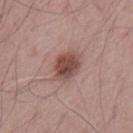The lesion was tiled from a total-body skin photograph and was not biopsied. A male subject, aged around 65. The total-body-photography lesion software estimated a footprint of about 10 mm², an eccentricity of roughly 0.7, and a shape-asymmetry score of about 0.2 (0 = symmetric). The software also gave an average lesion color of about L≈48 a*≈20 b*≈23 (CIELAB), roughly 13 lightness units darker than nearby skin, and a normalized lesion–skin contrast near 9. And it measured a border-irregularity rating of about 2/10, internal color variation of about 5 on a 0–10 scale, and a peripheral color-asymmetry measure near 1.5. And it measured a lesion-detection confidence of about 100/100. Located on the mid back. Imaged with white-light lighting. Cropped from a total-body skin-imaging series; the visible field is about 15 mm. Longest diameter approximately 4.5 mm.